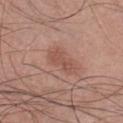follow-up = total-body-photography surveillance lesion; no biopsy
tile lighting = white-light illumination
lesion size = about 4 mm
patient = male, about 30 years old
location = the chest
imaging modality = ~15 mm tile from a whole-body skin photo